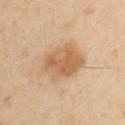The lesion was tiled from a total-body skin photograph and was not biopsied. Automated image analysis of the tile measured a lesion area of about 17 mm² and a shape eccentricity near 0.75. The software also gave internal color variation of about 4 on a 0–10 scale and a peripheral color-asymmetry measure near 1.5. About 5.5 mm across. A male patient, approximately 40 years of age. Cropped from a whole-body photographic skin survey; the tile spans about 15 mm. From the right upper arm. The tile uses cross-polarized illumination.